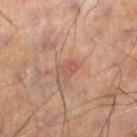Findings:
– biopsy status — no biopsy performed (imaged during a skin exam)
– site — the left lower leg
– lesion diameter — ≈3 mm
– tile lighting — cross-polarized illumination
– imaging modality — ~15 mm tile from a whole-body skin photo
– patient — male, aged around 60